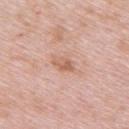Case summary:
– notes: total-body-photography surveillance lesion; no biopsy
– patient: female, aged approximately 65
– site: the upper back
– acquisition: ~15 mm crop, total-body skin-cancer survey